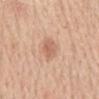Image and clinical context:
A 15 mm crop from a total-body photograph taken for skin-cancer surveillance. The total-body-photography lesion software estimated an area of roughly 9 mm², an outline eccentricity of about 0.8 (0 = round, 1 = elongated), and a symmetry-axis asymmetry near 0.2. The analysis additionally found border irregularity of about 2.5 on a 0–10 scale, a within-lesion color-variation index near 3/10, and peripheral color asymmetry of about 1. Captured under white-light illumination. From the back. The subject is a male aged around 60.